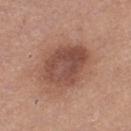No biopsy was performed on this lesion — it was imaged during a full skin examination and was not determined to be concerning. About 5.5 mm across. The lesion-visualizer software estimated an area of roughly 20 mm², a shape eccentricity near 0.6, and two-axis asymmetry of about 0.2. The patient is a female aged approximately 35. Located on the left thigh. A 15 mm close-up tile from a total-body photography series done for melanoma screening.